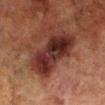patient = male, roughly 70 years of age
acquisition = ~15 mm crop, total-body skin-cancer survey
location = the right lower leg